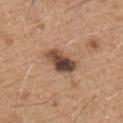Impression:
This lesion was catalogued during total-body skin photography and was not selected for biopsy.
Background:
This is a white-light tile. A region of skin cropped from a whole-body photographic capture, roughly 15 mm wide. Approximately 4 mm at its widest. A male subject aged 63 to 67. The lesion is located on the chest. The lesion-visualizer software estimated an area of roughly 7.5 mm² and a symmetry-axis asymmetry near 0.25. The analysis additionally found an average lesion color of about L≈45 a*≈19 b*≈27 (CIELAB), a lesion–skin lightness drop of about 17, and a normalized lesion–skin contrast near 12.5. The software also gave a classifier nevus-likeness of about 25/100 and lesion-presence confidence of about 100/100.